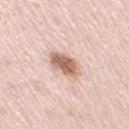<lesion>
<biopsy_status>not biopsied; imaged during a skin examination</biopsy_status>
<image>
  <source>total-body photography crop</source>
  <field_of_view_mm>15</field_of_view_mm>
</image>
<lighting>white-light</lighting>
<automated_metrics>
  <cielab_L>64</cielab_L>
  <cielab_a>19</cielab_a>
  <cielab_b>28</cielab_b>
  <vs_skin_darker_L>16.0</vs_skin_darker_L>
  <vs_skin_contrast_norm>9.5</vs_skin_contrast_norm>
  <border_irregularity_0_10>2.5</border_irregularity_0_10>
  <color_variation_0_10>4.0</color_variation_0_10>
  <nevus_likeness_0_100>95</nevus_likeness_0_100>
  <lesion_detection_confidence_0_100>100</lesion_detection_confidence_0_100>
</automated_metrics>
<lesion_size>
  <long_diameter_mm_approx>4.5</long_diameter_mm_approx>
</lesion_size>
<site>arm</site>
<patient>
  <sex>female</sex>
  <age_approx>65</age_approx>
</patient>
</lesion>